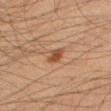  biopsy_status: not biopsied; imaged during a skin examination
  patient:
    sex: male
    age_approx: 35
  site: leg
  image:
    source: total-body photography crop
    field_of_view_mm: 15
  lighting: cross-polarized